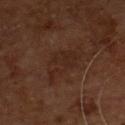– image-analysis metrics — a lesion area of about 12 mm², an outline eccentricity of about 0.9 (0 = round, 1 = elongated), and a shape-asymmetry score of about 0.4 (0 = symmetric)
– subject — male, aged approximately 55
– location — the chest
– imaging modality — total-body-photography crop, ~15 mm field of view
– size — ≈6 mm